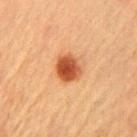Assessment: The lesion was photographed on a routine skin check and not biopsied; there is no pathology result. Acquisition and patient details: A roughly 15 mm field-of-view crop from a total-body skin photograph. A female subject, roughly 60 years of age. From the front of the torso.